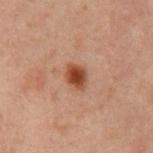Cropped from a total-body skin-imaging series; the visible field is about 15 mm.
Located on the chest.
A male subject, about 60 years old.